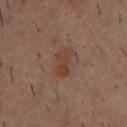<tbp_lesion>
<biopsy_status>not biopsied; imaged during a skin examination</biopsy_status>
<image>
  <source>total-body photography crop</source>
  <field_of_view_mm>15</field_of_view_mm>
</image>
<automated_metrics>
  <border_irregularity_0_10>2.0</border_irregularity_0_10>
  <color_variation_0_10>3.0</color_variation_0_10>
</automated_metrics>
<lesion_size>
  <long_diameter_mm_approx>3.5</long_diameter_mm_approx>
</lesion_size>
<site>back</site>
<patient>
  <sex>male</sex>
  <age_approx>40</age_approx>
</patient>
<lighting>cross-polarized</lighting>
</tbp_lesion>